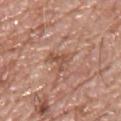The lesion was tiled from a total-body skin photograph and was not biopsied.
A male patient approximately 55 years of age.
Captured under white-light illumination.
A 15 mm close-up extracted from a 3D total-body photography capture.
The lesion-visualizer software estimated a lesion area of about 5 mm² and a shape eccentricity near 0.65. It also reported a lesion–skin lightness drop of about 9. And it measured peripheral color asymmetry of about 0.5. The software also gave an automated nevus-likeness rating near 0 out of 100 and a detector confidence of about 85 out of 100 that the crop contains a lesion.